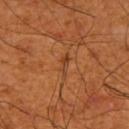Impression: Recorded during total-body skin imaging; not selected for excision or biopsy. Background: A region of skin cropped from a whole-body photographic capture, roughly 15 mm wide. Located on the arm. The patient is a male aged approximately 65.